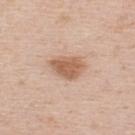biopsy status: no biopsy performed (imaged during a skin exam)
tile lighting: white-light
automated metrics: about 12 CIELAB-L* units darker than the surrounding skin and a lesion-to-skin contrast of about 8 (normalized; higher = more distinct); an automated nevus-likeness rating near 75 out of 100 and a detector confidence of about 100 out of 100 that the crop contains a lesion
anatomic site: the upper back
patient: male, aged 33–37
acquisition: ~15 mm crop, total-body skin-cancer survey
lesion size: ~4 mm (longest diameter)